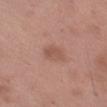notes=catalogued during a skin exam; not biopsied
lighting=white-light
subject=male, roughly 50 years of age
image source=15 mm crop, total-body photography
site=the leg
size=≈2.5 mm